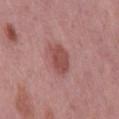This lesion was catalogued during total-body skin photography and was not selected for biopsy. From the mid back. This image is a 15 mm lesion crop taken from a total-body photograph. The patient is a male aged approximately 60.